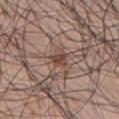The lesion was tiled from a total-body skin photograph and was not biopsied. Measured at roughly 2.5 mm in maximum diameter. The subject is a male aged 68–72. On the chest. A lesion tile, about 15 mm wide, cut from a 3D total-body photograph. This is a white-light tile.